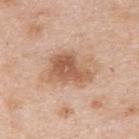Part of a total-body skin-imaging series; this lesion was reviewed on a skin check and was not flagged for biopsy. Imaged with white-light lighting. Located on the right upper arm. A female patient, approximately 45 years of age. A 15 mm close-up extracted from a 3D total-body photography capture. Approximately 6.5 mm at its widest.